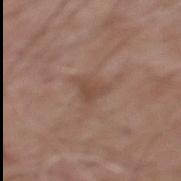workup: no biopsy performed (imaged during a skin exam); lesion diameter: ~3 mm (longest diameter); image: total-body-photography crop, ~15 mm field of view; lighting: white-light illumination; anatomic site: the mid back; subject: male, aged around 60.